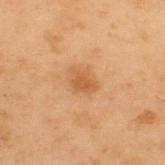{"biopsy_status": "not biopsied; imaged during a skin examination", "site": "upper back", "patient": {"sex": "male", "age_approx": 55}, "image": {"source": "total-body photography crop", "field_of_view_mm": 15}}